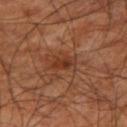{"biopsy_status": "not biopsied; imaged during a skin examination", "lighting": "cross-polarized", "lesion_size": {"long_diameter_mm_approx": 3.0}, "automated_metrics": {"area_mm2_approx": 4.0, "eccentricity": 0.85, "shape_asymmetry": 0.25}, "image": {"source": "total-body photography crop", "field_of_view_mm": 15}, "patient": {"sex": "male", "age_approx": 70}, "site": "right thigh"}